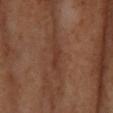Recorded during total-body skin imaging; not selected for excision or biopsy. The subject is female. The tile uses cross-polarized illumination. From the left forearm. About 2.5 mm across. A close-up tile cropped from a whole-body skin photograph, about 15 mm across.